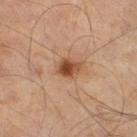The lesion was tiled from a total-body skin photograph and was not biopsied. An algorithmic analysis of the crop reported an outline eccentricity of about 0.65 (0 = round, 1 = elongated) and a shape-asymmetry score of about 0.2 (0 = symmetric). It also reported a border-irregularity rating of about 2/10, a within-lesion color-variation index near 5/10, and a peripheral color-asymmetry measure near 2. It also reported a nevus-likeness score of about 90/100. A male patient aged around 60. A lesion tile, about 15 mm wide, cut from a 3D total-body photograph. Imaged with cross-polarized lighting. Located on the right thigh. Approximately 3 mm at its widest.